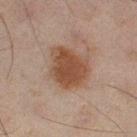This lesion was catalogued during total-body skin photography and was not selected for biopsy. A region of skin cropped from a whole-body photographic capture, roughly 15 mm wide. An algorithmic analysis of the crop reported an outline eccentricity of about 0.55 (0 = round, 1 = elongated) and two-axis asymmetry of about 0.25. And it measured an average lesion color of about L≈38 a*≈17 b*≈25 (CIELAB), about 9 CIELAB-L* units darker than the surrounding skin, and a normalized border contrast of about 9. The analysis additionally found a border-irregularity index near 2.5/10, internal color variation of about 3 on a 0–10 scale, and peripheral color asymmetry of about 1. Located on the left thigh. Captured under cross-polarized illumination. The recorded lesion diameter is about 5 mm. The subject is a male in their mid- to late 40s.